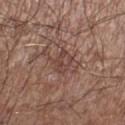Clinical impression: The lesion was tiled from a total-body skin photograph and was not biopsied. Acquisition and patient details: A male subject in their 60s. The lesion is located on the right lower leg. This is a white-light tile. Approximately 3 mm at its widest. A region of skin cropped from a whole-body photographic capture, roughly 15 mm wide.